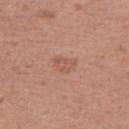Captured during whole-body skin photography for melanoma surveillance; the lesion was not biopsied. The lesion is located on the arm. A female patient, aged 53 to 57. The lesion's longest dimension is about 3 mm. A close-up tile cropped from a whole-body skin photograph, about 15 mm across.